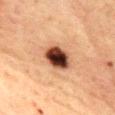notes: total-body-photography surveillance lesion; no biopsy | illumination: cross-polarized illumination | acquisition: total-body-photography crop, ~15 mm field of view | location: the front of the torso | patient: female, aged 58–62 | lesion diameter: ≈4 mm | automated lesion analysis: a lesion color around L≈36 a*≈20 b*≈27 in CIELAB and roughly 21 lightness units darker than nearby skin; a classifier nevus-likeness of about 100/100 and lesion-presence confidence of about 100/100.